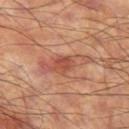body site = the left thigh | patient = male, about 60 years old | lesion diameter = ≈6 mm | image = ~15 mm tile from a whole-body skin photo.